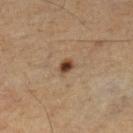Notes:
• follow-up: no biopsy performed (imaged during a skin exam)
• diameter: ~2 mm (longest diameter)
• location: the left lower leg
• illumination: cross-polarized illumination
• image: total-body-photography crop, ~15 mm field of view
• subject: male, aged around 55